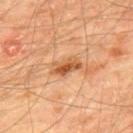Background:
Imaged with cross-polarized lighting. A region of skin cropped from a whole-body photographic capture, roughly 15 mm wide. Automated tile analysis of the lesion measured an area of roughly 6 mm², an eccentricity of roughly 0.85, and two-axis asymmetry of about 0.25. And it measured an average lesion color of about L≈56 a*≈25 b*≈40 (CIELAB), about 12 CIELAB-L* units darker than the surrounding skin, and a lesion-to-skin contrast of about 8 (normalized; higher = more distinct). The lesion is located on the mid back. Approximately 3.5 mm at its widest. A male patient, in their mid- to late 60s.